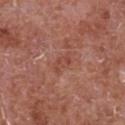  lighting: white-light
  site: chest
  automated_metrics:
    area_mm2_approx: 3.0
    eccentricity: 0.85
    shape_asymmetry: 0.45
    nevus_likeness_0_100: 0
    lesion_detection_confidence_0_100: 100
  image:
    source: total-body photography crop
    field_of_view_mm: 15
  patient:
    sex: male
    age_approx: 65